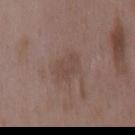About 3.5 mm across. This is a white-light tile. A region of skin cropped from a whole-body photographic capture, roughly 15 mm wide. The subject is a female in their mid- to late 30s. The lesion is located on the mid back.